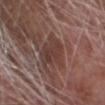Clinical impression:
The lesion was photographed on a routine skin check and not biopsied; there is no pathology result.
Context:
From the head or neck. The lesion-visualizer software estimated a footprint of about 10 mm² and a shape eccentricity near 0.55. And it measured a border-irregularity rating of about 3.5/10, a within-lesion color-variation index near 3.5/10, and peripheral color asymmetry of about 1. Longest diameter approximately 4 mm. This is a white-light tile. A 15 mm crop from a total-body photograph taken for skin-cancer surveillance. The patient is a male in their 70s.